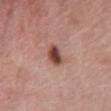Clinical summary: A roughly 15 mm field-of-view crop from a total-body skin photograph. From the chest. A female patient, in their mid-60s.